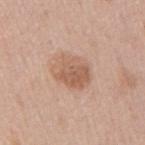The lesion was tiled from a total-body skin photograph and was not biopsied.
From the right upper arm.
A region of skin cropped from a whole-body photographic capture, roughly 15 mm wide.
A male patient, about 45 years old.